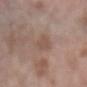Image and clinical context: The tile uses white-light illumination. Approximately 2.5 mm at its widest. The patient is a female aged 68 to 72. Automated image analysis of the tile measured an outline eccentricity of about 0.35 (0 = round, 1 = elongated) and two-axis asymmetry of about 0.3. And it measured a lesion color around L≈51 a*≈17 b*≈24 in CIELAB, a lesion–skin lightness drop of about 6, and a normalized lesion–skin contrast near 4.5. The software also gave a border-irregularity index near 2.5/10. The software also gave a nevus-likeness score of about 0/100 and a detector confidence of about 100 out of 100 that the crop contains a lesion. Cropped from a total-body skin-imaging series; the visible field is about 15 mm. On the left forearm.